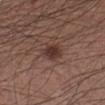Q: Was a biopsy performed?
A: no biopsy performed (imaged during a skin exam)
Q: What lighting was used for the tile?
A: white-light
Q: What kind of image is this?
A: total-body-photography crop, ~15 mm field of view
Q: What did automated image analysis measure?
A: a border-irregularity index near 1/10
Q: Who is the patient?
A: male, aged around 55
Q: Where on the body is the lesion?
A: the left forearm